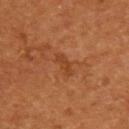Clinical impression:
The lesion was tiled from a total-body skin photograph and was not biopsied.
Acquisition and patient details:
About 3 mm across. The patient is a male aged 53–57. From the upper back. This image is a 15 mm lesion crop taken from a total-body photograph. Imaged with cross-polarized lighting.